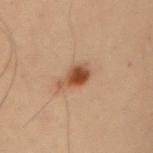Q: Was this lesion biopsied?
A: imaged on a skin check; not biopsied
Q: How was this image acquired?
A: ~15 mm crop, total-body skin-cancer survey
Q: What is the anatomic site?
A: the right upper arm
Q: Automated lesion metrics?
A: a lesion area of about 5 mm², a shape eccentricity near 0.7, and two-axis asymmetry of about 0.4; a within-lesion color-variation index near 3.5/10 and peripheral color asymmetry of about 1
Q: Lesion size?
A: about 3 mm
Q: Patient demographics?
A: male, aged 53–57